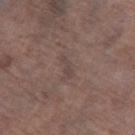Recorded during total-body skin imaging; not selected for excision or biopsy. Automated image analysis of the tile measured a mean CIELAB color near L≈43 a*≈14 b*≈18 and about 6 CIELAB-L* units darker than the surrounding skin. It also reported a classifier nevus-likeness of about 0/100 and lesion-presence confidence of about 60/100. The lesion's longest dimension is about 3 mm. The subject is a male in their mid- to late 70s. Imaged with white-light lighting. A 15 mm close-up extracted from a 3D total-body photography capture. The lesion is on the left thigh.